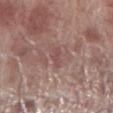Q: Is there a histopathology result?
A: catalogued during a skin exam; not biopsied
Q: What is the anatomic site?
A: the right lower leg
Q: What are the patient's age and sex?
A: male, roughly 70 years of age
Q: What is the imaging modality?
A: ~15 mm crop, total-body skin-cancer survey
Q: What did automated image analysis measure?
A: an average lesion color of about L≈49 a*≈20 b*≈20 (CIELAB) and roughly 7 lightness units darker than nearby skin; a classifier nevus-likeness of about 0/100 and a lesion-detection confidence of about 80/100